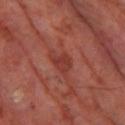This lesion was catalogued during total-body skin photography and was not selected for biopsy.
A male subject, aged 68–72.
The lesion is located on the left thigh.
A lesion tile, about 15 mm wide, cut from a 3D total-body photograph.